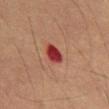| field | value |
|---|---|
| workup | total-body-photography surveillance lesion; no biopsy |
| image source | ~15 mm tile from a whole-body skin photo |
| site | the mid back |
| TBP lesion metrics | an area of roughly 4.5 mm², an outline eccentricity of about 0.65 (0 = round, 1 = elongated), and two-axis asymmetry of about 0.25; about 14 CIELAB-L* units darker than the surrounding skin and a normalized border contrast of about 12.5; a peripheral color-asymmetry measure near 1 |
| subject | male, aged approximately 55 |